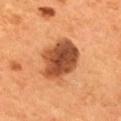notes = catalogued during a skin exam; not biopsied